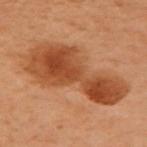Recorded during total-body skin imaging; not selected for excision or biopsy.
A female patient aged 58 to 62.
Cropped from a whole-body photographic skin survey; the tile spans about 15 mm.
The lesion is on the chest.
Captured under cross-polarized illumination.